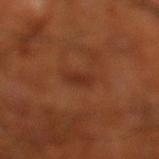Impression:
The lesion was tiled from a total-body skin photograph and was not biopsied.